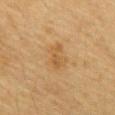Impression: The lesion was photographed on a routine skin check and not biopsied; there is no pathology result. Context: Located on the front of the torso. A male patient, aged approximately 85 or older. An algorithmic analysis of the crop reported a lesion area of about 6.5 mm², an eccentricity of roughly 0.8, and a symmetry-axis asymmetry near 0.3. The software also gave lesion-presence confidence of about 100/100. This image is a 15 mm lesion crop taken from a total-body photograph. The lesion's longest dimension is about 3.5 mm.